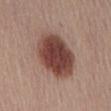The lesion was tiled from a total-body skin photograph and was not biopsied. Automated tile analysis of the lesion measured a footprint of about 24 mm², an outline eccentricity of about 0.7 (0 = round, 1 = elongated), and two-axis asymmetry of about 0.1. And it measured a mean CIELAB color near L≈43 a*≈22 b*≈23, a lesion–skin lightness drop of about 16, and a normalized lesion–skin contrast near 12. And it measured a classifier nevus-likeness of about 100/100 and a lesion-detection confidence of about 100/100. The recorded lesion diameter is about 6.5 mm. This is a white-light tile. The patient is a male about 55 years old. On the abdomen. A close-up tile cropped from a whole-body skin photograph, about 15 mm across.